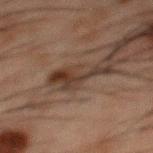<case>
  <biopsy_status>not biopsied; imaged during a skin examination</biopsy_status>
  <lighting>cross-polarized</lighting>
  <image>
    <source>total-body photography crop</source>
    <field_of_view_mm>15</field_of_view_mm>
  </image>
  <patient>
    <sex>male</sex>
    <age_approx>50</age_approx>
  </patient>
  <site>mid back</site>
</case>